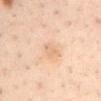Assessment:
Recorded during total-body skin imaging; not selected for excision or biopsy.
Background:
The patient is a male aged 38 to 42. Automated image analysis of the tile measured a border-irregularity index near 3/10 and radial color variation of about 1. A region of skin cropped from a whole-body photographic capture, roughly 15 mm wide. The tile uses cross-polarized illumination. The recorded lesion diameter is about 2.5 mm. The lesion is located on the mid back.